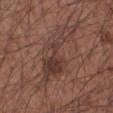No biopsy was performed on this lesion — it was imaged during a full skin examination and was not determined to be concerning. The recorded lesion diameter is about 8.5 mm. From the left forearm. A male subject roughly 55 years of age. A 15 mm crop from a total-body photograph taken for skin-cancer surveillance.